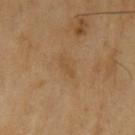Q: Was this lesion biopsied?
A: total-body-photography surveillance lesion; no biopsy
Q: What did automated image analysis measure?
A: a lesion area of about 2.5 mm² and an eccentricity of roughly 0.95; a border-irregularity index near 2.5/10, a color-variation rating of about 0/10, and peripheral color asymmetry of about 0
Q: What are the patient's age and sex?
A: male, about 70 years old
Q: What is the imaging modality?
A: 15 mm crop, total-body photography
Q: Lesion location?
A: the left upper arm
Q: What lighting was used for the tile?
A: cross-polarized